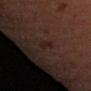Clinical impression: The lesion was photographed on a routine skin check and not biopsied; there is no pathology result. Image and clinical context: The patient is a female in their mid- to late 40s. A 15 mm close-up tile from a total-body photography series done for melanoma screening. Longest diameter approximately 2.5 mm. The tile uses cross-polarized illumination. The lesion is on the left forearm.